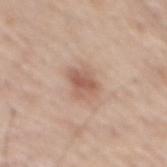follow-up: total-body-photography surveillance lesion; no biopsy | anatomic site: the back | tile lighting: white-light illumination | lesion size: ≈3 mm | subject: male, aged 63 to 67 | image: 15 mm crop, total-body photography.